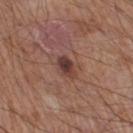This lesion was catalogued during total-body skin photography and was not selected for biopsy.
The total-body-photography lesion software estimated a footprint of about 4 mm² and a shape-asymmetry score of about 0.2 (0 = symmetric). It also reported an average lesion color of about L≈38 a*≈20 b*≈22 (CIELAB) and about 12 CIELAB-L* units darker than the surrounding skin. It also reported a border-irregularity rating of about 2/10, a color-variation rating of about 4/10, and radial color variation of about 1.5.
The lesion is located on the right thigh.
A male patient, roughly 55 years of age.
A lesion tile, about 15 mm wide, cut from a 3D total-body photograph.